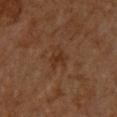Clinical impression:
The lesion was photographed on a routine skin check and not biopsied; there is no pathology result.
Acquisition and patient details:
This is a cross-polarized tile. On the upper back. The recorded lesion diameter is about 2.5 mm. The subject is a male approximately 60 years of age. Cropped from a whole-body photographic skin survey; the tile spans about 15 mm.